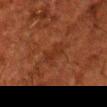– subject · male, about 60 years old
– lighting · cross-polarized
– image · ~15 mm crop, total-body skin-cancer survey
– body site · the head or neck
– automated metrics · a footprint of about 4.5 mm², an eccentricity of roughly 0.95, and a shape-asymmetry score of about 0.3 (0 = symmetric); a nevus-likeness score of about 0/100